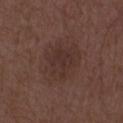Case summary:
* notes · no biopsy performed (imaged during a skin exam)
* illumination · white-light
* image source · 15 mm crop, total-body photography
* image-analysis metrics · a footprint of about 19 mm², a shape eccentricity near 0.45, and two-axis asymmetry of about 0.15; a lesion–skin lightness drop of about 5 and a normalized border contrast of about 5.5; a border-irregularity index near 2.5/10, a within-lesion color-variation index near 3/10, and radial color variation of about 1
* size · ~5.5 mm (longest diameter)
* subject · male, in their 50s